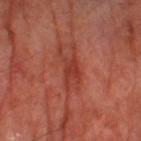<tbp_lesion>
<biopsy_status>not biopsied; imaged during a skin examination</biopsy_status>
<patient>
  <sex>male</sex>
  <age_approx>70</age_approx>
</patient>
<image>
  <source>total-body photography crop</source>
  <field_of_view_mm>15</field_of_view_mm>
</image>
<site>right thigh</site>
</tbp_lesion>